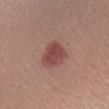follow-up = no biopsy performed (imaged during a skin exam); lesion diameter = ≈3.5 mm; lighting = white-light illumination; subject = female, roughly 25 years of age; imaging modality = ~15 mm tile from a whole-body skin photo; anatomic site = the right forearm.